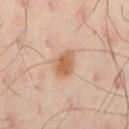Part of a total-body skin-imaging series; this lesion was reviewed on a skin check and was not flagged for biopsy. Located on the left thigh. The patient is a male roughly 45 years of age. A region of skin cropped from a whole-body photographic capture, roughly 15 mm wide.